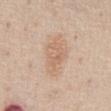  biopsy_status: not biopsied; imaged during a skin examination
  automated_metrics:
    area_mm2_approx: 13.0
    eccentricity: 0.9
    shape_asymmetry: 0.25
    cielab_L: 65
    cielab_a: 17
    cielab_b: 30
    vs_skin_contrast_norm: 5.5
    nevus_likeness_0_100: 5
  patient:
    sex: male
    age_approx: 60
  image:
    source: total-body photography crop
    field_of_view_mm: 15
  site: abdomen
  lesion_size:
    long_diameter_mm_approx: 6.5
  lighting: white-light